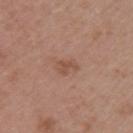{
  "biopsy_status": "not biopsied; imaged during a skin examination",
  "automated_metrics": {
    "nevus_likeness_0_100": 0,
    "lesion_detection_confidence_0_100": 100
  },
  "lighting": "white-light",
  "patient": {
    "sex": "female",
    "age_approx": 45
  },
  "lesion_size": {
    "long_diameter_mm_approx": 2.5
  },
  "site": "left upper arm",
  "image": {
    "source": "total-body photography crop",
    "field_of_view_mm": 15
  }
}